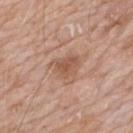follow-up = imaged on a skin check; not biopsied | site = the mid back | size = ~4 mm (longest diameter) | patient = male, roughly 60 years of age | image source = 15 mm crop, total-body photography.